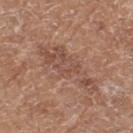Recorded during total-body skin imaging; not selected for excision or biopsy.
The lesion is located on the left thigh.
Captured under white-light illumination.
The lesion's longest dimension is about 8 mm.
The patient is a female aged approximately 75.
A lesion tile, about 15 mm wide, cut from a 3D total-body photograph.
The total-body-photography lesion software estimated an area of roughly 15 mm², a shape eccentricity near 0.95, and a symmetry-axis asymmetry near 0.4. The software also gave border irregularity of about 8 on a 0–10 scale and radial color variation of about 1.